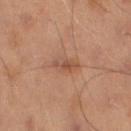The lesion was photographed on a routine skin check and not biopsied; there is no pathology result.
On the left thigh.
A roughly 15 mm field-of-view crop from a total-body skin photograph.
Approximately 3 mm at its widest.
Automated tile analysis of the lesion measured a border-irregularity rating of about 4/10, a color-variation rating of about 0.5/10, and a peripheral color-asymmetry measure near 0. The analysis additionally found a lesion-detection confidence of about 100/100.
The tile uses cross-polarized illumination.
A male subject, aged approximately 70.